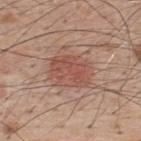notes: total-body-photography surveillance lesion; no biopsy | imaging modality: total-body-photography crop, ~15 mm field of view | patient: male, about 50 years old | location: the upper back | lighting: white-light illumination.